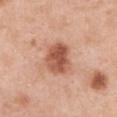• follow-up — catalogued during a skin exam; not biopsied
• body site — the chest
• lesion size — ~4 mm (longest diameter)
• subject — female, aged approximately 40
• tile lighting — white-light
• image source — total-body-photography crop, ~15 mm field of view